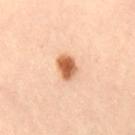lesion diameter — ~3 mm (longest diameter)
body site — the lower back
patient — female, roughly 40 years of age
image — ~15 mm crop, total-body skin-cancer survey